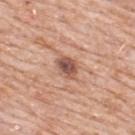Imaged during a routine full-body skin examination; the lesion was not biopsied and no histopathology is available. On the back. Approximately 3 mm at its widest. Imaged with white-light lighting. A lesion tile, about 15 mm wide, cut from a 3D total-body photograph. The patient is a male roughly 80 years of age. An algorithmic analysis of the crop reported a border-irregularity rating of about 2/10 and radial color variation of about 1.5. The software also gave a detector confidence of about 100 out of 100 that the crop contains a lesion.